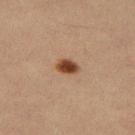<lesion>
  <biopsy_status>not biopsied; imaged during a skin examination</biopsy_status>
  <lesion_size>
    <long_diameter_mm_approx>2.5</long_diameter_mm_approx>
  </lesion_size>
  <patient>
    <sex>female</sex>
    <age_approx>30</age_approx>
  </patient>
  <site>left thigh</site>
  <automated_metrics>
    <cielab_L>39</cielab_L>
    <cielab_a>20</cielab_a>
    <cielab_b>30</cielab_b>
    <vs_skin_contrast_norm>12.5</vs_skin_contrast_norm>
    <border_irregularity_0_10>1.5</border_irregularity_0_10>
    <peripheral_color_asymmetry>1.0</peripheral_color_asymmetry>
  </automated_metrics>
  <image>
    <source>total-body photography crop</source>
    <field_of_view_mm>15</field_of_view_mm>
  </image>
  <lighting>cross-polarized</lighting>
</lesion>